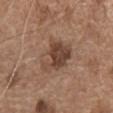workup = no biopsy performed (imaged during a skin exam)
acquisition = ~15 mm tile from a whole-body skin photo
location = the chest
tile lighting = white-light
lesion diameter = ~4.5 mm (longest diameter)
patient = male, about 75 years old